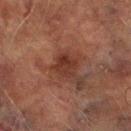Recorded during total-body skin imaging; not selected for excision or biopsy. Cropped from a whole-body photographic skin survey; the tile spans about 15 mm. A male patient, aged approximately 75. The recorded lesion diameter is about 3 mm. This is a cross-polarized tile. Automated tile analysis of the lesion measured an average lesion color of about L≈28 a*≈20 b*≈23 (CIELAB), a lesion–skin lightness drop of about 7, and a lesion-to-skin contrast of about 7.5 (normalized; higher = more distinct). The software also gave an automated nevus-likeness rating near 5 out of 100 and a lesion-detection confidence of about 100/100. Located on the right lower leg.